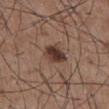The lesion's longest dimension is about 3 mm.
From the abdomen.
The patient is a male in their mid- to late 50s.
Cropped from a total-body skin-imaging series; the visible field is about 15 mm.
The tile uses white-light illumination.
The total-body-photography lesion software estimated a lesion color around L≈37 a*≈18 b*≈23 in CIELAB, about 13 CIELAB-L* units darker than the surrounding skin, and a normalized lesion–skin contrast near 11. The software also gave a border-irregularity rating of about 1.5/10, a within-lesion color-variation index near 5/10, and radial color variation of about 1.5. The analysis additionally found a classifier nevus-likeness of about 95/100 and a detector confidence of about 100 out of 100 that the crop contains a lesion.